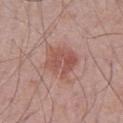Impression: Captured during whole-body skin photography for melanoma surveillance; the lesion was not biopsied. Context: Cropped from a total-body skin-imaging series; the visible field is about 15 mm. The subject is a male roughly 70 years of age. Longest diameter approximately 5 mm. The tile uses white-light illumination. The lesion is located on the abdomen.